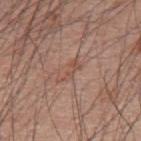Impression: This lesion was catalogued during total-body skin photography and was not selected for biopsy. Acquisition and patient details: The lesion is on the upper back. The lesion's longest dimension is about 3.5 mm. Automated tile analysis of the lesion measured a border-irregularity index near 6/10, a within-lesion color-variation index near 0.5/10, and peripheral color asymmetry of about 0. A region of skin cropped from a whole-body photographic capture, roughly 15 mm wide. A male patient, in their 60s. This is a white-light tile.